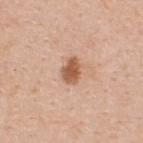– biopsy status — total-body-photography surveillance lesion; no biopsy
– location — the upper back
– acquisition — ~15 mm tile from a whole-body skin photo
– lighting — white-light
– subject — female, aged around 30
– lesion size — about 3 mm